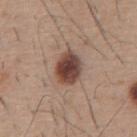Clinical impression: Imaged during a routine full-body skin examination; the lesion was not biopsied and no histopathology is available. Background: The tile uses white-light illumination. Cropped from a whole-body photographic skin survey; the tile spans about 15 mm. A male subject, about 60 years old. On the abdomen. An algorithmic analysis of the crop reported a lesion area of about 10 mm², a shape eccentricity near 0.6, and two-axis asymmetry of about 0.15. The software also gave an automated nevus-likeness rating near 100 out of 100 and a detector confidence of about 100 out of 100 that the crop contains a lesion. The recorded lesion diameter is about 4 mm.